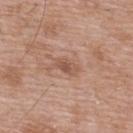No biopsy was performed on this lesion — it was imaged during a full skin examination and was not determined to be concerning. A male subject roughly 50 years of age. A 15 mm close-up tile from a total-body photography series done for melanoma screening. The total-body-photography lesion software estimated an average lesion color of about L≈53 a*≈21 b*≈28 (CIELAB), about 9 CIELAB-L* units darker than the surrounding skin, and a normalized border contrast of about 6.5. The software also gave a border-irregularity index near 2/10 and radial color variation of about 0.5. This is a white-light tile. On the upper back. About 3 mm across.